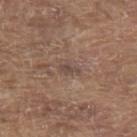Captured during whole-body skin photography for melanoma surveillance; the lesion was not biopsied.
The lesion is on the upper back.
The lesion's longest dimension is about 3 mm.
A roughly 15 mm field-of-view crop from a total-body skin photograph.
A male subject in their 80s.
Imaged with white-light lighting.